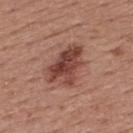Clinical impression:
No biopsy was performed on this lesion — it was imaged during a full skin examination and was not determined to be concerning.
Background:
The lesion's longest dimension is about 5.5 mm. From the upper back. An algorithmic analysis of the crop reported an area of roughly 13 mm², a shape eccentricity near 0.75, and two-axis asymmetry of about 0.35. The software also gave a border-irregularity index near 4.5/10 and radial color variation of about 2. A roughly 15 mm field-of-view crop from a total-body skin photograph. The subject is a male aged 53–57. This is a white-light tile.